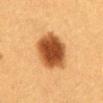patient:
  sex: female
  age_approx: 40
site: abdomen
image:
  source: total-body photography crop
  field_of_view_mm: 15